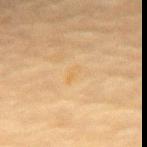<tbp_lesion>
<image>
  <source>total-body photography crop</source>
  <field_of_view_mm>15</field_of_view_mm>
</image>
<site>chest</site>
<patient>
  <sex>female</sex>
  <age_approx>80</age_approx>
</patient>
<lesion_size>
  <long_diameter_mm_approx>2.5</long_diameter_mm_approx>
</lesion_size>
<lighting>cross-polarized</lighting>
</tbp_lesion>